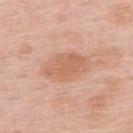Q: Is there a histopathology result?
A: total-body-photography surveillance lesion; no biopsy
Q: What lighting was used for the tile?
A: white-light illumination
Q: Automated lesion metrics?
A: a footprint of about 13 mm², a shape eccentricity near 0.8, and two-axis asymmetry of about 0.15; border irregularity of about 2 on a 0–10 scale, a within-lesion color-variation index near 2.5/10, and a peripheral color-asymmetry measure near 1; an automated nevus-likeness rating near 0 out of 100 and a detector confidence of about 100 out of 100 that the crop contains a lesion
Q: What kind of image is this?
A: ~15 mm crop, total-body skin-cancer survey
Q: Lesion location?
A: the upper back
Q: What is the lesion's diameter?
A: ~5.5 mm (longest diameter)
Q: What are the patient's age and sex?
A: female, in their 50s